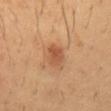No biopsy was performed on this lesion — it was imaged during a full skin examination and was not determined to be concerning. On the abdomen. A close-up tile cropped from a whole-body skin photograph, about 15 mm across. A male subject, roughly 50 years of age. The tile uses cross-polarized illumination.